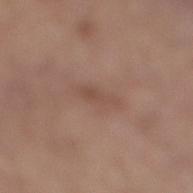follow-up — catalogued during a skin exam; not biopsied | TBP lesion metrics — an average lesion color of about L≈49 a*≈18 b*≈26 (CIELAB), a lesion–skin lightness drop of about 6, and a normalized border contrast of about 5; radial color variation of about 0; an automated nevus-likeness rating near 0 out of 100 | lighting — white-light illumination | site — the leg | acquisition — total-body-photography crop, ~15 mm field of view | lesion size — ≈3.5 mm | patient — female, aged around 65.